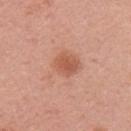Q: Was a biopsy performed?
A: no biopsy performed (imaged during a skin exam)
Q: How was this image acquired?
A: 15 mm crop, total-body photography
Q: What are the patient's age and sex?
A: female, approximately 50 years of age
Q: What is the lesion's diameter?
A: ~4.5 mm (longest diameter)
Q: Where on the body is the lesion?
A: the left upper arm
Q: What lighting was used for the tile?
A: white-light illumination